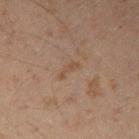follow-up: catalogued during a skin exam; not biopsied
acquisition: ~15 mm crop, total-body skin-cancer survey
diameter: about 3 mm
patient: male, aged 48–52
site: the right upper arm
tile lighting: cross-polarized illumination